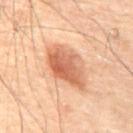Case summary:
• notes · total-body-photography surveillance lesion; no biopsy
• subject · male, aged around 70
• size · ≈5.5 mm
• lighting · cross-polarized
• acquisition · ~15 mm tile from a whole-body skin photo
• site · the abdomen